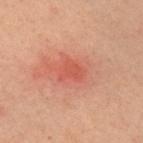<case>
<site>right upper arm</site>
<patient>
  <sex>male</sex>
  <age_approx>60</age_approx>
</patient>
<image>
  <source>total-body photography crop</source>
  <field_of_view_mm>15</field_of_view_mm>
</image>
<lesion_size>
  <long_diameter_mm_approx>2.5</long_diameter_mm_approx>
</lesion_size>
<lighting>cross-polarized</lighting>
</case>